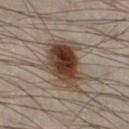The lesion was tiled from a total-body skin photograph and was not biopsied.
The patient is a male aged 48 to 52.
Approximately 5.5 mm at its widest.
Imaged with cross-polarized lighting.
The lesion is on the leg.
A roughly 15 mm field-of-view crop from a total-body skin photograph.